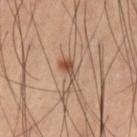workup: no biopsy performed (imaged during a skin exam)
automated metrics: an area of roughly 4.5 mm², a shape eccentricity near 0.75, and a shape-asymmetry score of about 0.4 (0 = symmetric); an average lesion color of about L≈50 a*≈18 b*≈29 (CIELAB) and a lesion-to-skin contrast of about 7.5 (normalized; higher = more distinct); a color-variation rating of about 6/10; an automated nevus-likeness rating near 95 out of 100 and a lesion-detection confidence of about 100/100
location: the left thigh
subject: male, approximately 60 years of age
tile lighting: cross-polarized
acquisition: total-body-photography crop, ~15 mm field of view
lesion size: about 3 mm